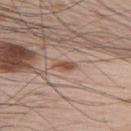Automated tile analysis of the lesion measured an average lesion color of about L≈51 a*≈20 b*≈28 (CIELAB), roughly 10 lightness units darker than nearby skin, and a lesion-to-skin contrast of about 8 (normalized; higher = more distinct). And it measured a nevus-likeness score of about 85/100. Approximately 2.5 mm at its widest. A male patient, roughly 65 years of age. A roughly 15 mm field-of-view crop from a total-body skin photograph. Located on the upper back. The tile uses white-light illumination.